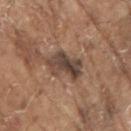Notes:
- notes: no biopsy performed (imaged during a skin exam)
- image-analysis metrics: an automated nevus-likeness rating near 0 out of 100 and a detector confidence of about 85 out of 100 that the crop contains a lesion
- lighting: white-light illumination
- acquisition: 15 mm crop, total-body photography
- body site: the right upper arm
- lesion size: ≈5 mm
- subject: male, aged 78–82